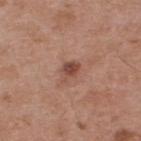The lesion was tiled from a total-body skin photograph and was not biopsied. From the upper back. The subject is a male about 55 years old. Captured under white-light illumination. A lesion tile, about 15 mm wide, cut from a 3D total-body photograph. Approximately 3 mm at its widest.